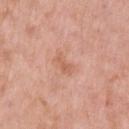Clinical impression: Imaged during a routine full-body skin examination; the lesion was not biopsied and no histopathology is available. Context: From the arm. A close-up tile cropped from a whole-body skin photograph, about 15 mm across. An algorithmic analysis of the crop reported a lesion color around L≈61 a*≈24 b*≈32 in CIELAB, about 7 CIELAB-L* units darker than the surrounding skin, and a lesion-to-skin contrast of about 5 (normalized; higher = more distinct). It also reported internal color variation of about 2.5 on a 0–10 scale and a peripheral color-asymmetry measure near 1. Imaged with white-light lighting. A male subject approximately 50 years of age. Longest diameter approximately 3 mm.